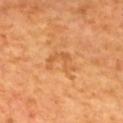Impression:
No biopsy was performed on this lesion — it was imaged during a full skin examination and was not determined to be concerning.
Context:
From the upper back. Longest diameter approximately 4 mm. The patient is a male aged around 65. A lesion tile, about 15 mm wide, cut from a 3D total-body photograph. Imaged with cross-polarized lighting.